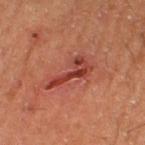Impression:
No biopsy was performed on this lesion — it was imaged during a full skin examination and was not determined to be concerning.
Acquisition and patient details:
A 15 mm close-up extracted from a 3D total-body photography capture. Measured at roughly 6 mm in maximum diameter. From the right lower leg. A male patient, in their mid-50s. Imaged with cross-polarized lighting.